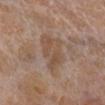| feature | finding |
|---|---|
| follow-up | catalogued during a skin exam; not biopsied |